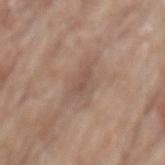Assessment:
The lesion was tiled from a total-body skin photograph and was not biopsied.
Context:
A close-up tile cropped from a whole-body skin photograph, about 15 mm across. A male subject, in their 80s. The lesion's longest dimension is about 3 mm. On the mid back.